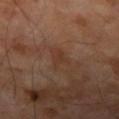TBP lesion metrics: border irregularity of about 5 on a 0–10 scale and peripheral color asymmetry of about 0.5; a classifier nevus-likeness of about 0/100 and a detector confidence of about 100 out of 100 that the crop contains a lesion | image: ~15 mm crop, total-body skin-cancer survey | site: the right forearm | subject: male, aged around 70 | tile lighting: cross-polarized | diameter: ≈3 mm.